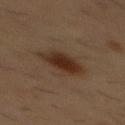{"biopsy_status": "not biopsied; imaged during a skin examination", "image": {"source": "total-body photography crop", "field_of_view_mm": 15}, "patient": {"sex": "male", "age_approx": 55}, "site": "chest", "automated_metrics": {"vs_skin_contrast_norm": 10.0, "peripheral_color_asymmetry": 1.0}}